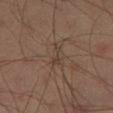This lesion was catalogued during total-body skin photography and was not selected for biopsy.
An algorithmic analysis of the crop reported an average lesion color of about L≈35 a*≈14 b*≈21 (CIELAB) and about 5 CIELAB-L* units darker than the surrounding skin. It also reported a border-irregularity rating of about 4.5/10, internal color variation of about 0 on a 0–10 scale, and a peripheral color-asymmetry measure near 0. The software also gave a nevus-likeness score of about 0/100 and lesion-presence confidence of about 55/100.
A male subject, approximately 35 years of age.
Cropped from a whole-body photographic skin survey; the tile spans about 15 mm.
This is a cross-polarized tile.
The lesion is located on the left thigh.
Longest diameter approximately 3 mm.